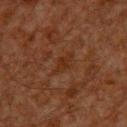{"biopsy_status": "not biopsied; imaged during a skin examination", "site": "back", "lesion_size": {"long_diameter_mm_approx": 3.0}, "patient": {"sex": "male", "age_approx": 60}, "lighting": "cross-polarized", "image": {"source": "total-body photography crop", "field_of_view_mm": 15}}